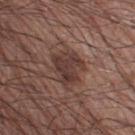The lesion was tiled from a total-body skin photograph and was not biopsied. A roughly 15 mm field-of-view crop from a total-body skin photograph. The lesion is located on the right thigh. A male subject about 60 years old.